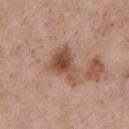Q: Is there a histopathology result?
A: catalogued during a skin exam; not biopsied
Q: What is the lesion's diameter?
A: ≈5 mm
Q: Where on the body is the lesion?
A: the front of the torso
Q: What is the imaging modality?
A: total-body-photography crop, ~15 mm field of view
Q: Patient demographics?
A: male, about 55 years old
Q: What lighting was used for the tile?
A: white-light
Q: What did automated image analysis measure?
A: a shape eccentricity near 0.8 and a shape-asymmetry score of about 0.4 (0 = symmetric); a mean CIELAB color near L≈50 a*≈21 b*≈29, a lesion–skin lightness drop of about 12, and a normalized border contrast of about 9; a within-lesion color-variation index near 6.5/10 and peripheral color asymmetry of about 2